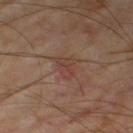| field | value |
|---|---|
| workup | total-body-photography surveillance lesion; no biopsy |
| subject | male, in their mid- to late 60s |
| tile lighting | cross-polarized illumination |
| anatomic site | the right thigh |
| automated metrics | an area of roughly 5 mm², a shape eccentricity near 0.4, and two-axis asymmetry of about 0.4; a border-irregularity rating of about 3.5/10 and peripheral color asymmetry of about 1.5; an automated nevus-likeness rating near 0 out of 100 |
| diameter | ≈2.5 mm |
| image | 15 mm crop, total-body photography |